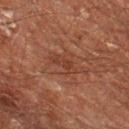Notes:
• follow-up · no biopsy performed (imaged during a skin exam)
• location · the left thigh
• TBP lesion metrics · a footprint of about 3 mm²; a border-irregularity rating of about 5/10, a color-variation rating of about 0/10, and radial color variation of about 0; a classifier nevus-likeness of about 0/100 and a lesion-detection confidence of about 95/100
• imaging modality · ~15 mm crop, total-body skin-cancer survey
• lesion diameter · ~3 mm (longest diameter)
• patient · male, about 60 years old
• lighting · cross-polarized illumination